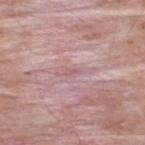* notes · total-body-photography surveillance lesion; no biopsy
* illumination · white-light
* automated metrics · a mean CIELAB color near L≈58 a*≈24 b*≈17, a lesion–skin lightness drop of about 7, and a lesion-to-skin contrast of about 4.5 (normalized; higher = more distinct); a classifier nevus-likeness of about 0/100 and a detector confidence of about 10 out of 100 that the crop contains a lesion
* site · the upper back
* imaging modality · total-body-photography crop, ~15 mm field of view
* subject · male, aged around 55